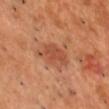Clinical impression: Captured during whole-body skin photography for melanoma surveillance; the lesion was not biopsied. Context: A male patient about 50 years old. Located on the chest. Imaged with cross-polarized lighting. A roughly 15 mm field-of-view crop from a total-body skin photograph. Automated image analysis of the tile measured a normalized border contrast of about 6. And it measured a border-irregularity index near 3.5/10 and a color-variation rating of about 2.5/10.